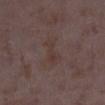<lesion>
  <biopsy_status>not biopsied; imaged during a skin examination</biopsy_status>
  <lighting>white-light</lighting>
  <site>right lower leg</site>
  <patient>
    <sex>female</sex>
    <age_approx>35</age_approx>
  </patient>
  <lesion_size>
    <long_diameter_mm_approx>4.5</long_diameter_mm_approx>
  </lesion_size>
  <image>
    <source>total-body photography crop</source>
    <field_of_view_mm>15</field_of_view_mm>
  </image>
</lesion>